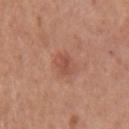Background: On the left upper arm. A female subject, roughly 45 years of age. This image is a 15 mm lesion crop taken from a total-body photograph. This is a white-light tile. The lesion's longest dimension is about 3 mm. The total-body-photography lesion software estimated an area of roughly 4.5 mm², an outline eccentricity of about 0.7 (0 = round, 1 = elongated), and a symmetry-axis asymmetry near 0.2. And it measured a classifier nevus-likeness of about 30/100 and lesion-presence confidence of about 100/100.